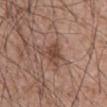workup = no biopsy performed (imaged during a skin exam) | acquisition = 15 mm crop, total-body photography | lesion size = ~3 mm (longest diameter) | patient = male, roughly 60 years of age | body site = the abdomen | lighting = white-light illumination.